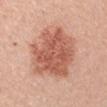Q: Was this lesion biopsied?
A: imaged on a skin check; not biopsied
Q: Where on the body is the lesion?
A: the left upper arm
Q: Patient demographics?
A: male, about 40 years old
Q: How was this image acquired?
A: ~15 mm crop, total-body skin-cancer survey
Q: What is the lesion's diameter?
A: ~7.5 mm (longest diameter)
Q: Illumination type?
A: white-light
Q: What did automated image analysis measure?
A: a mean CIELAB color near L≈58 a*≈26 b*≈31, about 13 CIELAB-L* units darker than the surrounding skin, and a normalized lesion–skin contrast near 8.5; lesion-presence confidence of about 100/100